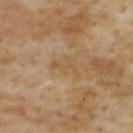<case>
<biopsy_status>not biopsied; imaged during a skin examination</biopsy_status>
<patient>
  <sex>female</sex>
  <age_approx>60</age_approx>
</patient>
<image>
  <source>total-body photography crop</source>
  <field_of_view_mm>15</field_of_view_mm>
</image>
<site>upper back</site>
</case>